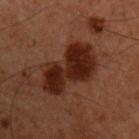workup — no biopsy performed (imaged during a skin exam) | anatomic site — the front of the torso | imaging modality — total-body-photography crop, ~15 mm field of view | size — about 7.5 mm | image-analysis metrics — a footprint of about 20 mm² and a shape eccentricity near 0.9; roughly 10 lightness units darker than nearby skin; border irregularity of about 4.5 on a 0–10 scale, a color-variation rating of about 3/10, and radial color variation of about 1; an automated nevus-likeness rating near 75 out of 100 and a detector confidence of about 100 out of 100 that the crop contains a lesion | subject — male, aged 58 to 62.